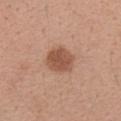Part of a total-body skin-imaging series; this lesion was reviewed on a skin check and was not flagged for biopsy. A roughly 15 mm field-of-view crop from a total-body skin photograph. Located on the arm. A female subject, aged around 40.